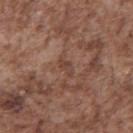biopsy status: total-body-photography surveillance lesion; no biopsy | diameter: ~2.5 mm (longest diameter) | lighting: white-light illumination | location: the back | image: ~15 mm tile from a whole-body skin photo | patient: male, about 55 years old | automated metrics: a border-irregularity index near 3.5/10 and a peripheral color-asymmetry measure near 0; a nevus-likeness score of about 0/100 and a lesion-detection confidence of about 95/100.